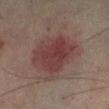The lesion is on the left lower leg. About 6 mm across. The patient is a male roughly 75 years of age. A roughly 15 mm field-of-view crop from a total-body skin photograph. The tile uses cross-polarized illumination.